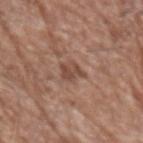Recorded during total-body skin imaging; not selected for excision or biopsy. A male patient aged approximately 70. Automated tile analysis of the lesion measured roughly 9 lightness units darker than nearby skin and a lesion-to-skin contrast of about 7 (normalized; higher = more distinct). The analysis additionally found a border-irregularity index near 3.5/10, a within-lesion color-variation index near 3/10, and a peripheral color-asymmetry measure near 1.5. It also reported lesion-presence confidence of about 100/100. Cropped from a total-body skin-imaging series; the visible field is about 15 mm. Captured under white-light illumination. The lesion's longest dimension is about 2.5 mm. The lesion is located on the left forearm.